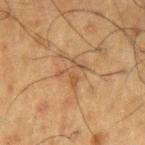| feature | finding |
|---|---|
| lighting | cross-polarized illumination |
| imaging modality | ~15 mm crop, total-body skin-cancer survey |
| subject | male, in their 60s |
| site | the left upper arm |